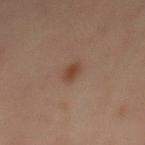<tbp_lesion>
  <biopsy_status>not biopsied; imaged during a skin examination</biopsy_status>
  <patient>
    <sex>male</sex>
    <age_approx>40</age_approx>
  </patient>
  <automated_metrics>
    <cielab_L>33</cielab_L>
    <cielab_a>16</cielab_a>
    <cielab_b>23</cielab_b>
    <vs_skin_darker_L>7.0</vs_skin_darker_L>
    <border_irregularity_0_10>1.5</border_irregularity_0_10>
    <color_variation_0_10>1.0</color_variation_0_10>
    <peripheral_color_asymmetry>0.5</peripheral_color_asymmetry>
    <nevus_likeness_0_100>95</nevus_likeness_0_100>
    <lesion_detection_confidence_0_100>100</lesion_detection_confidence_0_100>
  </automated_metrics>
  <lighting>cross-polarized</lighting>
  <site>mid back</site>
  <image>
    <source>total-body photography crop</source>
    <field_of_view_mm>15</field_of_view_mm>
  </image>
</tbp_lesion>